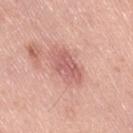No biopsy was performed on this lesion — it was imaged during a full skin examination and was not determined to be concerning. Measured at roughly 5.5 mm in maximum diameter. Automated tile analysis of the lesion measured an area of roughly 11 mm², an eccentricity of roughly 0.85, and a symmetry-axis asymmetry near 0.15. The software also gave border irregularity of about 2.5 on a 0–10 scale, internal color variation of about 3 on a 0–10 scale, and peripheral color asymmetry of about 1. The software also gave an automated nevus-likeness rating near 5 out of 100. Located on the lower back. A close-up tile cropped from a whole-body skin photograph, about 15 mm across. This is a white-light tile. A female subject, about 40 years old.